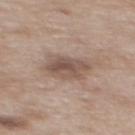Notes:
- tile lighting: white-light
- diameter: ~4.5 mm (longest diameter)
- image: ~15 mm crop, total-body skin-cancer survey
- location: the upper back
- patient: female, aged around 40